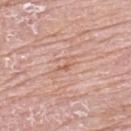notes: catalogued during a skin exam; not biopsied | anatomic site: the upper back | TBP lesion metrics: an eccentricity of roughly 0.95 and a symmetry-axis asymmetry near 0.65; an average lesion color of about L≈62 a*≈22 b*≈30 (CIELAB), roughly 7 lightness units darker than nearby skin, and a lesion-to-skin contrast of about 5.5 (normalized; higher = more distinct); a border-irregularity index near 7.5/10, a within-lesion color-variation index near 0/10, and peripheral color asymmetry of about 0; a classifier nevus-likeness of about 0/100 and a detector confidence of about 85 out of 100 that the crop contains a lesion | tile lighting: white-light | subject: female, approximately 65 years of age | image: ~15 mm tile from a whole-body skin photo.